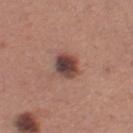notes: total-body-photography surveillance lesion; no biopsy
image source: total-body-photography crop, ~15 mm field of view
anatomic site: the left thigh
subject: female, about 30 years old
tile lighting: white-light illumination